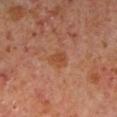Q: Was a biopsy performed?
A: total-body-photography surveillance lesion; no biopsy
Q: What did automated image analysis measure?
A: an average lesion color of about L≈44 a*≈24 b*≈33 (CIELAB) and roughly 6 lightness units darker than nearby skin
Q: What lighting was used for the tile?
A: cross-polarized
Q: What kind of image is this?
A: ~15 mm crop, total-body skin-cancer survey
Q: What is the lesion's diameter?
A: about 2.5 mm
Q: Where on the body is the lesion?
A: the leg
Q: What are the patient's age and sex?
A: male, aged approximately 60